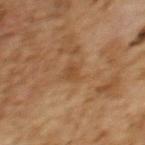follow-up=no biopsy performed (imaged during a skin exam)
patient=female, aged 58–62
site=the upper back
image source=total-body-photography crop, ~15 mm field of view
size=~2.5 mm (longest diameter)